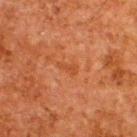Recorded during total-body skin imaging; not selected for excision or biopsy.
Located on the upper back.
Cropped from a whole-body photographic skin survey; the tile spans about 15 mm.
The subject is a male in their 60s.